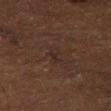| key | value |
|---|---|
| biopsy status | catalogued during a skin exam; not biopsied |
| size | about 3 mm |
| TBP lesion metrics | a footprint of about 3 mm², an eccentricity of roughly 0.9, and a symmetry-axis asymmetry near 0.35; a mean CIELAB color near L≈20 a*≈12 b*≈16, about 3 CIELAB-L* units darker than the surrounding skin, and a lesion-to-skin contrast of about 5 (normalized; higher = more distinct); a detector confidence of about 70 out of 100 that the crop contains a lesion |
| patient | male, about 75 years old |
| body site | the right thigh |
| acquisition | total-body-photography crop, ~15 mm field of view |